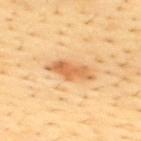follow-up: total-body-photography surveillance lesion; no biopsy | subject: male, in their mid-40s | image-analysis metrics: a lesion color around L≈64 a*≈24 b*≈44 in CIELAB, a lesion–skin lightness drop of about 12, and a normalized border contrast of about 7.5; a border-irregularity index near 3.5/10, a within-lesion color-variation index near 3.5/10, and radial color variation of about 1.5 | anatomic site: the upper back | lesion size: ~5 mm (longest diameter) | tile lighting: cross-polarized illumination | acquisition: ~15 mm crop, total-body skin-cancer survey.